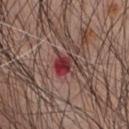A roughly 15 mm field-of-view crop from a total-body skin photograph.
The patient is a male in their 60s.
On the chest.
About 3 mm across.